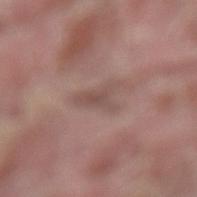Assessment: The lesion was tiled from a total-body skin photograph and was not biopsied. Acquisition and patient details: A 15 mm crop from a total-body photograph taken for skin-cancer surveillance. This is a white-light tile. A male patient aged 38 to 42. Measured at roughly 4.5 mm in maximum diameter. The lesion is on the back. Automated tile analysis of the lesion measured a mean CIELAB color near L≈49 a*≈18 b*≈21, roughly 7 lightness units darker than nearby skin, and a lesion-to-skin contrast of about 5 (normalized; higher = more distinct). It also reported a classifier nevus-likeness of about 0/100.